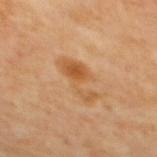This lesion was catalogued during total-body skin photography and was not selected for biopsy. On the upper back. Measured at roughly 5 mm in maximum diameter. This image is a 15 mm lesion crop taken from a total-body photograph. A female subject, in their mid- to late 60s. Imaged with cross-polarized lighting.